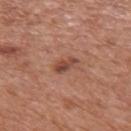Recorded during total-body skin imaging; not selected for excision or biopsy.
A male subject, aged 68–72.
The lesion is on the mid back.
Longest diameter approximately 3 mm.
This is a white-light tile.
A 15 mm close-up extracted from a 3D total-body photography capture.
Automated tile analysis of the lesion measured a border-irregularity index near 2.5/10 and a within-lesion color-variation index near 3/10.